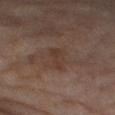The lesion was tiled from a total-body skin photograph and was not biopsied. A roughly 15 mm field-of-view crop from a total-body skin photograph. On the right thigh. An algorithmic analysis of the crop reported a shape eccentricity near 0.65 and two-axis asymmetry of about 0.3. The software also gave a mean CIELAB color near L≈32 a*≈15 b*≈21, roughly 5 lightness units darker than nearby skin, and a lesion-to-skin contrast of about 5.5 (normalized; higher = more distinct). The analysis additionally found a border-irregularity rating of about 3/10, a color-variation rating of about 2/10, and peripheral color asymmetry of about 1. The lesion's longest dimension is about 2.5 mm. A female patient approximately 60 years of age. This is a cross-polarized tile.